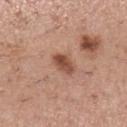lighting: white-light illumination | anatomic site: the leg | TBP lesion metrics: a lesion area of about 5 mm², an eccentricity of roughly 0.75, and a shape-asymmetry score of about 0.25 (0 = symmetric); a lesion color around L≈50 a*≈23 b*≈29 in CIELAB and a lesion–skin lightness drop of about 12; a border-irregularity index near 2/10, internal color variation of about 4.5 on a 0–10 scale, and a peripheral color-asymmetry measure near 1.5 | acquisition: ~15 mm crop, total-body skin-cancer survey | subject: female, approximately 30 years of age.